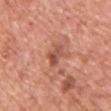Assessment:
The lesion was tiled from a total-body skin photograph and was not biopsied.
Image and clinical context:
The lesion is on the chest. A female patient roughly 40 years of age. Cropped from a total-body skin-imaging series; the visible field is about 15 mm.